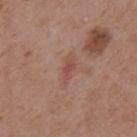Recorded during total-body skin imaging; not selected for excision or biopsy. A roughly 15 mm field-of-view crop from a total-body skin photograph. A male subject, aged around 55. The lesion is located on the back. An algorithmic analysis of the crop reported a mean CIELAB color near L≈49 a*≈25 b*≈24, about 7 CIELAB-L* units darker than the surrounding skin, and a normalized lesion–skin contrast near 5.5.